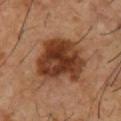notes: no biopsy performed (imaged during a skin exam); acquisition: total-body-photography crop, ~15 mm field of view; patient: male, aged 53 to 57; automated lesion analysis: a lesion color around L≈36 a*≈22 b*≈31 in CIELAB and a lesion–skin lightness drop of about 15; size: ≈6.5 mm; anatomic site: the chest.